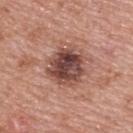No biopsy was performed on this lesion — it was imaged during a full skin examination and was not determined to be concerning.
The lesion-visualizer software estimated a mean CIELAB color near L≈46 a*≈22 b*≈24 and a lesion–skin lightness drop of about 15. It also reported border irregularity of about 2.5 on a 0–10 scale, internal color variation of about 8 on a 0–10 scale, and radial color variation of about 3.
The lesion's longest dimension is about 4.5 mm.
A female subject, in their 60s.
The lesion is on the upper back.
The tile uses white-light illumination.
A 15 mm close-up tile from a total-body photography series done for melanoma screening.